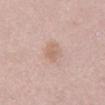• biopsy status: catalogued during a skin exam; not biopsied
• body site: the mid back
• diameter: about 3 mm
• acquisition: ~15 mm tile from a whole-body skin photo
• patient: female, in their mid-60s
• illumination: white-light illumination